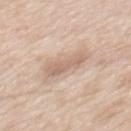Notes:
* biopsy status — total-body-photography surveillance lesion; no biopsy
* image — ~15 mm tile from a whole-body skin photo
* tile lighting — white-light illumination
* subject — male, aged 58–62
* body site — the mid back